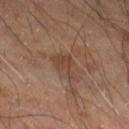{
  "biopsy_status": "not biopsied; imaged during a skin examination",
  "site": "right thigh",
  "lesion_size": {
    "long_diameter_mm_approx": 3.5
  },
  "image": {
    "source": "total-body photography crop",
    "field_of_view_mm": 15
  },
  "patient": {
    "sex": "male",
    "age_approx": 55
  },
  "automated_metrics": {
    "border_irregularity_0_10": 5.5,
    "color_variation_0_10": 1.5,
    "peripheral_color_asymmetry": 0.5,
    "lesion_detection_confidence_0_100": 100
  },
  "lighting": "cross-polarized"
}